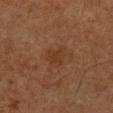| field | value |
|---|---|
| workup | catalogued during a skin exam; not biopsied |
| subject | male, approximately 80 years of age |
| lesion diameter | ~3 mm (longest diameter) |
| site | the front of the torso |
| lighting | cross-polarized |
| acquisition | 15 mm crop, total-body photography |